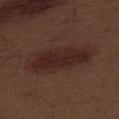Impression: This lesion was catalogued during total-body skin photography and was not selected for biopsy. Clinical summary: This is a white-light tile. Cropped from a total-body skin-imaging series; the visible field is about 15 mm. A male subject, roughly 70 years of age. About 8.5 mm across. The lesion is located on the leg.